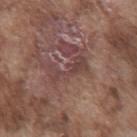Imaged during a routine full-body skin examination; the lesion was not biopsied and no histopathology is available. The tile uses white-light illumination. The lesion is on the front of the torso. Measured at roughly 4.5 mm in maximum diameter. A roughly 15 mm field-of-view crop from a total-body skin photograph. Automated tile analysis of the lesion measured a normalized lesion–skin contrast near 6. The analysis additionally found a lesion-detection confidence of about 50/100. A male subject, approximately 75 years of age.